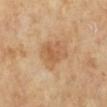Part of a total-body skin-imaging series; this lesion was reviewed on a skin check and was not flagged for biopsy.
The tile uses cross-polarized illumination.
A roughly 15 mm field-of-view crop from a total-body skin photograph.
Longest diameter approximately 4.5 mm.
Located on the left lower leg.
The patient is a female aged around 70.